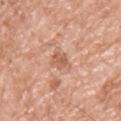Recorded during total-body skin imaging; not selected for excision or biopsy.